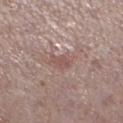Notes:
• workup · catalogued during a skin exam; not biopsied
• diameter · ≈2.5 mm
• acquisition · ~15 mm crop, total-body skin-cancer survey
• automated lesion analysis · an area of roughly 3.5 mm², an eccentricity of roughly 0.8, and two-axis asymmetry of about 0.35; an average lesion color of about L≈52 a*≈19 b*≈22 (CIELAB), about 8 CIELAB-L* units darker than the surrounding skin, and a lesion-to-skin contrast of about 5.5 (normalized; higher = more distinct); a color-variation rating of about 1/10 and peripheral color asymmetry of about 0.5; an automated nevus-likeness rating near 0 out of 100
• subject · male, approximately 70 years of age
• illumination · white-light
• body site · the left lower leg